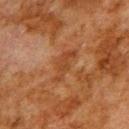{"biopsy_status": "not biopsied; imaged during a skin examination", "automated_metrics": {"area_mm2_approx": 6.5, "eccentricity": 0.9, "shape_asymmetry": 0.35, "cielab_L": 34, "cielab_a": 20, "cielab_b": 30, "vs_skin_darker_L": 6.0, "vs_skin_contrast_norm": 6.0, "border_irregularity_0_10": 4.5, "color_variation_0_10": 1.5, "peripheral_color_asymmetry": 0.5}, "lesion_size": {"long_diameter_mm_approx": 4.5}, "patient": {"sex": "male", "age_approx": 80}, "lighting": "cross-polarized", "site": "upper back", "image": {"source": "total-body photography crop", "field_of_view_mm": 15}}